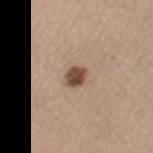| feature | finding |
|---|---|
| notes | total-body-photography surveillance lesion; no biopsy |
| image | 15 mm crop, total-body photography |
| illumination | white-light |
| subject | female, about 60 years old |
| size | ~2.5 mm (longest diameter) |
| body site | the leg |
| automated metrics | a symmetry-axis asymmetry near 0.25; an automated nevus-likeness rating near 95 out of 100 and lesion-presence confidence of about 100/100 |